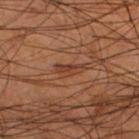Imaged during a routine full-body skin examination; the lesion was not biopsied and no histopathology is available. A lesion tile, about 15 mm wide, cut from a 3D total-body photograph. Measured at roughly 3.5 mm in maximum diameter. Automated tile analysis of the lesion measured a symmetry-axis asymmetry near 0.4. And it measured an average lesion color of about L≈36 a*≈22 b*≈28 (CIELAB), about 7 CIELAB-L* units darker than the surrounding skin, and a lesion-to-skin contrast of about 6.5 (normalized; higher = more distinct). It also reported a border-irregularity rating of about 4.5/10 and a color-variation rating of about 0.5/10. The patient is a male approximately 55 years of age. From the left lower leg.